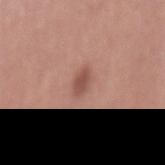biopsy status: imaged on a skin check; not biopsied | patient: female, aged 48 to 52 | site: the mid back | lesion size: ≈3 mm | acquisition: 15 mm crop, total-body photography.